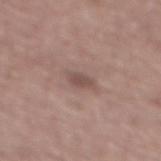Assessment: No biopsy was performed on this lesion — it was imaged during a full skin examination and was not determined to be concerning. Context: On the back. The subject is a male aged 58 to 62. About 2.5 mm across. The lesion-visualizer software estimated a border-irregularity index near 2.5/10, internal color variation of about 1.5 on a 0–10 scale, and a peripheral color-asymmetry measure near 0.5. The software also gave a nevus-likeness score of about 0/100 and lesion-presence confidence of about 100/100. Cropped from a whole-body photographic skin survey; the tile spans about 15 mm. The tile uses white-light illumination.